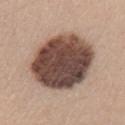Impression: The lesion was photographed on a routine skin check and not biopsied; there is no pathology result. Context: Captured under white-light illumination. A 15 mm close-up extracted from a 3D total-body photography capture. Located on the left upper arm. A female subject approximately 40 years of age.